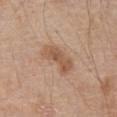Assessment:
The lesion was tiled from a total-body skin photograph and was not biopsied.
Clinical summary:
The subject is a male aged 78–82. On the abdomen. The tile uses white-light illumination. The total-body-photography lesion software estimated a mean CIELAB color near L≈55 a*≈19 b*≈31, roughly 9 lightness units darker than nearby skin, and a normalized lesion–skin contrast near 7. Cropped from a total-body skin-imaging series; the visible field is about 15 mm.